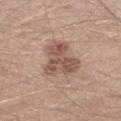* workup: imaged on a skin check; not biopsied
* automated metrics: a footprint of about 14 mm², a shape eccentricity near 0.7, and a symmetry-axis asymmetry near 0.5; a mean CIELAB color near L≈53 a*≈18 b*≈26, roughly 12 lightness units darker than nearby skin, and a normalized border contrast of about 8; a classifier nevus-likeness of about 30/100 and a detector confidence of about 100 out of 100 that the crop contains a lesion
* location: the left thigh
* subject: male, aged 28–32
* tile lighting: white-light illumination
* imaging modality: 15 mm crop, total-body photography